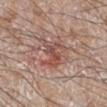Clinical impression: Imaged during a routine full-body skin examination; the lesion was not biopsied and no histopathology is available. Background: The total-body-photography lesion software estimated an area of roughly 9 mm², an outline eccentricity of about 0.4 (0 = round, 1 = elongated), and two-axis asymmetry of about 0.35. It also reported a mean CIELAB color near L≈51 a*≈22 b*≈25 and a normalized lesion–skin contrast near 6.5. Located on the right lower leg. Longest diameter approximately 4 mm. This is a white-light tile. A male subject, aged 58–62. A 15 mm close-up tile from a total-body photography series done for melanoma screening.